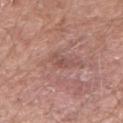Recorded during total-body skin imaging; not selected for excision or biopsy. Measured at roughly 2.5 mm in maximum diameter. The lesion is on the right forearm. This is a white-light tile. A male patient about 60 years old. A roughly 15 mm field-of-view crop from a total-body skin photograph. The lesion-visualizer software estimated a footprint of about 3.5 mm², a shape eccentricity near 0.75, and two-axis asymmetry of about 0.35. It also reported a lesion color around L≈51 a*≈22 b*≈24 in CIELAB and a normalized border contrast of about 5. It also reported a nevus-likeness score of about 0/100 and lesion-presence confidence of about 100/100.